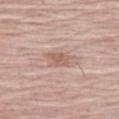• image source: ~15 mm tile from a whole-body skin photo
• lesion diameter: ~2.5 mm (longest diameter)
• subject: female, aged 63–67
• TBP lesion metrics: an area of roughly 4 mm², an eccentricity of roughly 0.7, and two-axis asymmetry of about 0.3; a border-irregularity rating of about 3/10
• location: the left thigh
• tile lighting: white-light illumination